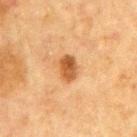Q: Was a biopsy performed?
A: catalogued during a skin exam; not biopsied
Q: Lesion location?
A: the chest
Q: What is the imaging modality?
A: ~15 mm tile from a whole-body skin photo
Q: What is the lesion's diameter?
A: about 3 mm
Q: What lighting was used for the tile?
A: cross-polarized illumination
Q: What are the patient's age and sex?
A: male, about 65 years old
Q: Automated lesion metrics?
A: a lesion color around L≈45 a*≈21 b*≈36 in CIELAB and a normalized lesion–skin contrast near 9; a border-irregularity rating of about 1.5/10 and a color-variation rating of about 4.5/10; a classifier nevus-likeness of about 90/100